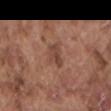{"biopsy_status": "not biopsied; imaged during a skin examination", "lesion_size": {"long_diameter_mm_approx": 3.0}, "patient": {"sex": "male", "age_approx": 75}, "image": {"source": "total-body photography crop", "field_of_view_mm": 15}, "lighting": "white-light", "site": "mid back"}